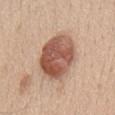follow-up: catalogued during a skin exam; not biopsied
subject: male, aged 58 to 62
location: the chest
image: total-body-photography crop, ~15 mm field of view